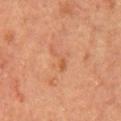Imaged during a routine full-body skin examination; the lesion was not biopsied and no histopathology is available. Imaged with cross-polarized lighting. The recorded lesion diameter is about 3.5 mm. The lesion-visualizer software estimated a border-irregularity index near 7.5/10, a color-variation rating of about 0/10, and a peripheral color-asymmetry measure near 0. And it measured an automated nevus-likeness rating near 0 out of 100 and lesion-presence confidence of about 100/100. A 15 mm close-up extracted from a 3D total-body photography capture. The subject is a male aged 63 to 67. The lesion is located on the chest.